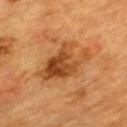– body site · the upper back
– patient · female, aged 53–57
– lesion size · ~6.5 mm (longest diameter)
– acquisition · ~15 mm crop, total-body skin-cancer survey